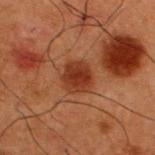Assessment: This lesion was catalogued during total-body skin photography and was not selected for biopsy.